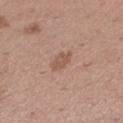<tbp_lesion>
<biopsy_status>not biopsied; imaged during a skin examination</biopsy_status>
<image>
  <source>total-body photography crop</source>
  <field_of_view_mm>15</field_of_view_mm>
</image>
<patient>
  <sex>female</sex>
  <age_approx>30</age_approx>
</patient>
<site>left lower leg</site>
<lesion_size>
  <long_diameter_mm_approx>3.0</long_diameter_mm_approx>
</lesion_size>
<automated_metrics>
  <cielab_L>54</cielab_L>
  <cielab_a>20</cielab_a>
  <cielab_b>27</cielab_b>
  <vs_skin_contrast_norm>6.0</vs_skin_contrast_norm>
  <nevus_likeness_0_100>20</nevus_likeness_0_100>
</automated_metrics>
</tbp_lesion>